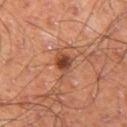Captured during whole-body skin photography for melanoma surveillance; the lesion was not biopsied.
A lesion tile, about 15 mm wide, cut from a 3D total-body photograph.
Located on the left thigh.
A male patient, about 60 years old.